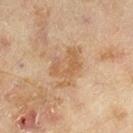Assessment:
Imaged during a routine full-body skin examination; the lesion was not biopsied and no histopathology is available.
Context:
Located on the right lower leg. A female patient, aged approximately 60. A 15 mm crop from a total-body photograph taken for skin-cancer surveillance. The total-body-photography lesion software estimated a border-irregularity rating of about 5/10 and a color-variation rating of about 3.5/10. The analysis additionally found a classifier nevus-likeness of about 0/100 and lesion-presence confidence of about 100/100. About 5 mm across.